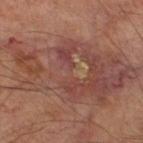Clinical impression:
The lesion was tiled from a total-body skin photograph and was not biopsied.
Background:
The lesion is located on the right thigh. A lesion tile, about 15 mm wide, cut from a 3D total-body photograph. A male subject approximately 70 years of age. Imaged with cross-polarized lighting.